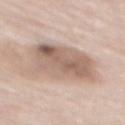<tbp_lesion>
  <biopsy_status>not biopsied; imaged during a skin examination</biopsy_status>
  <patient>
    <sex>male</sex>
    <age_approx>85+</age_approx>
  </patient>
  <image>
    <source>total-body photography crop</source>
    <field_of_view_mm>15</field_of_view_mm>
  </image>
  <site>mid back</site>
</tbp_lesion>